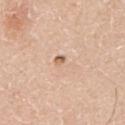The lesion was photographed on a routine skin check and not biopsied; there is no pathology result. The lesion is located on the front of the torso. This is a white-light tile. A 15 mm close-up tile from a total-body photography series done for melanoma screening. A male patient approximately 60 years of age.